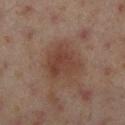workup — catalogued during a skin exam; not biopsied | lesion size — ~4.5 mm (longest diameter) | acquisition — total-body-photography crop, ~15 mm field of view | tile lighting — cross-polarized | anatomic site — the right lower leg | automated metrics — a lesion area of about 13 mm², a shape eccentricity near 0.5, and two-axis asymmetry of about 0.3; about 8 CIELAB-L* units darker than the surrounding skin and a lesion-to-skin contrast of about 6.5 (normalized; higher = more distinct); a within-lesion color-variation index near 3.5/10 and peripheral color asymmetry of about 1 | subject — female, roughly 40 years of age.